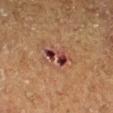• notes — no biopsy performed (imaged during a skin exam)
• imaging modality — 15 mm crop, total-body photography
• diameter — ~4 mm (longest diameter)
• subject — male, in their mid- to late 70s
• automated lesion analysis — a shape eccentricity near 0.85; border irregularity of about 4 on a 0–10 scale, a within-lesion color-variation index near 10/10, and peripheral color asymmetry of about 4.5; an automated nevus-likeness rating near 0 out of 100 and lesion-presence confidence of about 100/100
• illumination — cross-polarized
• site — the right lower leg